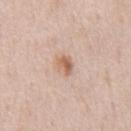Q: Was this lesion biopsied?
A: catalogued during a skin exam; not biopsied
Q: What kind of image is this?
A: ~15 mm tile from a whole-body skin photo
Q: Lesion location?
A: the chest
Q: Patient demographics?
A: male, in their 50s